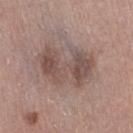Context: A roughly 15 mm field-of-view crop from a total-body skin photograph. About 6.5 mm across. From the leg. A female patient aged 63–67. Captured under white-light illumination. The lesion-visualizer software estimated an area of roughly 19 mm² and a symmetry-axis asymmetry near 0.35. The analysis additionally found border irregularity of about 6.5 on a 0–10 scale, a within-lesion color-variation index near 4.5/10, and radial color variation of about 1.5. It also reported a classifier nevus-likeness of about 15/100 and a lesion-detection confidence of about 100/100.